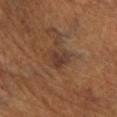biopsy status: total-body-photography surveillance lesion; no biopsy
automated metrics: a shape eccentricity near 0.55; a lesion color around L≈34 a*≈18 b*≈25 in CIELAB, roughly 7 lightness units darker than nearby skin, and a lesion-to-skin contrast of about 7 (normalized; higher = more distinct); a detector confidence of about 100 out of 100 that the crop contains a lesion
tile lighting: cross-polarized
size: ~3 mm (longest diameter)
subject: female, aged approximately 65
image: ~15 mm crop, total-body skin-cancer survey
location: the right lower leg